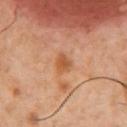– notes · imaged on a skin check; not biopsied
– acquisition · ~15 mm tile from a whole-body skin photo
– patient · male, about 55 years old
– body site · the chest
– image-analysis metrics · a footprint of about 4.5 mm², a shape eccentricity near 0.65, and a symmetry-axis asymmetry near 0.35
– lesion size · ≈2.5 mm
– tile lighting · cross-polarized